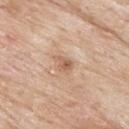On the back. A 15 mm close-up extracted from a 3D total-body photography capture. A male subject, roughly 85 years of age or older.